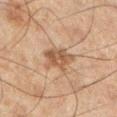Q: How was this image acquired?
A: total-body-photography crop, ~15 mm field of view
Q: What are the patient's age and sex?
A: male, aged approximately 45
Q: Automated lesion metrics?
A: an eccentricity of roughly 0.75; a mean CIELAB color near L≈44 a*≈16 b*≈28 and a lesion-to-skin contrast of about 7.5 (normalized; higher = more distinct); lesion-presence confidence of about 100/100
Q: What is the anatomic site?
A: the left lower leg